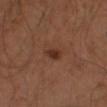follow-up: total-body-photography surveillance lesion; no biopsy
subject: male, aged 43–47
image source: ~15 mm tile from a whole-body skin photo
lighting: cross-polarized illumination
body site: the arm
TBP lesion metrics: a lesion color around L≈29 a*≈20 b*≈26 in CIELAB, roughly 9 lightness units darker than nearby skin, and a lesion-to-skin contrast of about 8.5 (normalized; higher = more distinct); a border-irregularity rating of about 2.5/10 and a color-variation rating of about 2/10